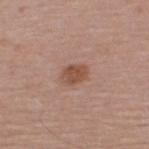Part of a total-body skin-imaging series; this lesion was reviewed on a skin check and was not flagged for biopsy.
This image is a 15 mm lesion crop taken from a total-body photograph.
A male subject, aged around 40.
The lesion is located on the upper back.